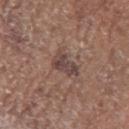acquisition: total-body-photography crop, ~15 mm field of view
TBP lesion metrics: an average lesion color of about L≈43 a*≈17 b*≈20 (CIELAB), a lesion–skin lightness drop of about 9, and a lesion-to-skin contrast of about 8 (normalized; higher = more distinct)
site: the right forearm
tile lighting: white-light illumination
subject: female, roughly 75 years of age